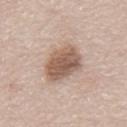The lesion was tiled from a total-body skin photograph and was not biopsied.
About 4.5 mm across.
The subject is a male about 65 years old.
Located on the back.
A close-up tile cropped from a whole-body skin photograph, about 15 mm across.